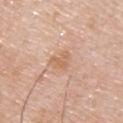Findings:
• workup — catalogued during a skin exam; not biopsied
• anatomic site — the back
• acquisition — 15 mm crop, total-body photography
• patient — male, aged 48–52